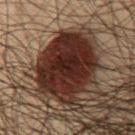Acquisition and patient details:
The lesion is on the front of the torso. A male patient, aged approximately 50. Longest diameter approximately 9 mm. A lesion tile, about 15 mm wide, cut from a 3D total-body photograph. Imaged with cross-polarized lighting.